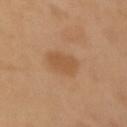follow-up = total-body-photography surveillance lesion; no biopsy
image source = ~15 mm tile from a whole-body skin photo
lesion size = ~4 mm (longest diameter)
illumination = cross-polarized illumination
patient = female, aged approximately 55
site = the left upper arm